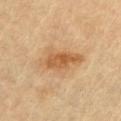Q: Was this lesion biopsied?
A: total-body-photography surveillance lesion; no biopsy
Q: How large is the lesion?
A: about 5 mm
Q: What did automated image analysis measure?
A: a within-lesion color-variation index near 4.5/10 and radial color variation of about 1.5; a classifier nevus-likeness of about 70/100 and a lesion-detection confidence of about 100/100
Q: What lighting was used for the tile?
A: cross-polarized illumination
Q: How was this image acquired?
A: ~15 mm tile from a whole-body skin photo
Q: What are the patient's age and sex?
A: male, aged 83 or older
Q: Where on the body is the lesion?
A: the chest